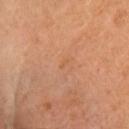{"lesion_size": {"long_diameter_mm_approx": 1.5}, "lighting": "cross-polarized", "image": {"source": "total-body photography crop", "field_of_view_mm": 15}, "automated_metrics": {"cielab_L": 59, "cielab_a": 24, "cielab_b": 39, "vs_skin_darker_L": 4.0, "vs_skin_contrast_norm": 4.0}, "patient": {"sex": "female", "age_approx": 60}, "site": "head or neck"}